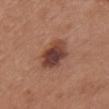This lesion was catalogued during total-body skin photography and was not selected for biopsy. The recorded lesion diameter is about 4.5 mm. Imaged with white-light lighting. From the front of the torso. A 15 mm close-up tile from a total-body photography series done for melanoma screening. A female subject roughly 65 years of age.